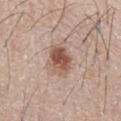Q: Was a biopsy performed?
A: catalogued during a skin exam; not biopsied
Q: How was this image acquired?
A: 15 mm crop, total-body photography
Q: Lesion size?
A: ≈4 mm
Q: Automated lesion metrics?
A: an area of roughly 9 mm², an outline eccentricity of about 0.75 (0 = round, 1 = elongated), and a symmetry-axis asymmetry near 0.15; border irregularity of about 1.5 on a 0–10 scale and a within-lesion color-variation index near 6.5/10
Q: Patient demographics?
A: male, about 65 years old
Q: Illumination type?
A: white-light illumination
Q: What is the anatomic site?
A: the abdomen